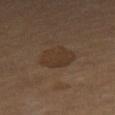The tile uses cross-polarized illumination. A female subject about 70 years old. A 15 mm close-up tile from a total-body photography series done for melanoma screening. The total-body-photography lesion software estimated an area of roughly 10 mm². The analysis additionally found an average lesion color of about L≈32 a*≈14 b*≈25 (CIELAB) and a normalized lesion–skin contrast near 6. The software also gave an automated nevus-likeness rating near 35 out of 100 and a detector confidence of about 100 out of 100 that the crop contains a lesion. The lesion is located on the abdomen. Measured at roughly 4 mm in maximum diameter.